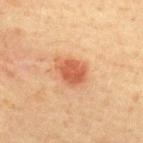| field | value |
|---|---|
| workup | imaged on a skin check; not biopsied |
| image | ~15 mm crop, total-body skin-cancer survey |
| size | ≈4 mm |
| subject | female, about 40 years old |
| body site | the upper back |
| tile lighting | cross-polarized illumination |